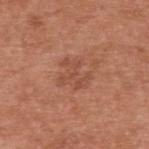<case>
  <biopsy_status>not biopsied; imaged during a skin examination</biopsy_status>
  <image>
    <source>total-body photography crop</source>
    <field_of_view_mm>15</field_of_view_mm>
  </image>
  <lighting>white-light</lighting>
  <lesion_size>
    <long_diameter_mm_approx>3.5</long_diameter_mm_approx>
  </lesion_size>
  <site>upper back</site>
  <patient>
    <sex>male</sex>
    <age_approx>55</age_approx>
  </patient>
</case>